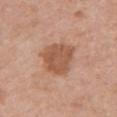Findings:
- follow-up: catalogued during a skin exam; not biopsied
- location: the chest
- acquisition: total-body-photography crop, ~15 mm field of view
- subject: female, aged 58 to 62
- tile lighting: white-light
- image-analysis metrics: a mean CIELAB color near L≈54 a*≈23 b*≈32 and a normalized lesion–skin contrast near 8; a border-irregularity index near 2.5/10 and a color-variation rating of about 2.5/10; an automated nevus-likeness rating near 40 out of 100
- size: ≈4.5 mm